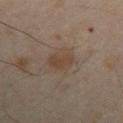The lesion was tiled from a total-body skin photograph and was not biopsied. An algorithmic analysis of the crop reported a lesion area of about 6.5 mm², an eccentricity of roughly 0.75, and two-axis asymmetry of about 0.25. The software also gave a mean CIELAB color near L≈37 a*≈13 b*≈23 and a lesion-to-skin contrast of about 6.5 (normalized; higher = more distinct). It also reported a border-irregularity rating of about 2.5/10, a color-variation rating of about 1.5/10, and radial color variation of about 0.5. And it measured a nevus-likeness score of about 20/100 and lesion-presence confidence of about 100/100. A 15 mm crop from a total-body photograph taken for skin-cancer surveillance. The subject is a male aged 48 to 52. Approximately 3.5 mm at its widest. From the left upper arm.